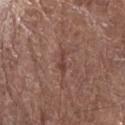follow-up — total-body-photography surveillance lesion; no biopsy | image — ~15 mm tile from a whole-body skin photo | lighting — white-light | lesion diameter — about 2.5 mm | anatomic site — the right forearm | patient — female, about 80 years old.